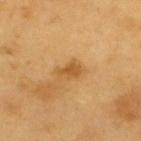Assessment: This lesion was catalogued during total-body skin photography and was not selected for biopsy. Background: The patient is a male aged around 60. A 15 mm crop from a total-body photograph taken for skin-cancer surveillance. The lesion is located on the upper back. About 2.5 mm across.